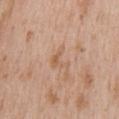Impression: Imaged during a routine full-body skin examination; the lesion was not biopsied and no histopathology is available. Image and clinical context: A male subject, in their mid-60s. The lesion is located on the front of the torso. A region of skin cropped from a whole-body photographic capture, roughly 15 mm wide. About 3 mm across.